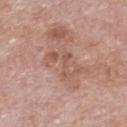Captured during whole-body skin photography for melanoma surveillance; the lesion was not biopsied. A 15 mm close-up tile from a total-body photography series done for melanoma screening. The subject is a male roughly 55 years of age. Located on the chest. Automated image analysis of the tile measured a lesion area of about 6 mm², an eccentricity of roughly 0.8, and a shape-asymmetry score of about 0.75 (0 = symmetric). The software also gave a color-variation rating of about 1/10 and peripheral color asymmetry of about 0. And it measured a classifier nevus-likeness of about 0/100 and a detector confidence of about 100 out of 100 that the crop contains a lesion. The tile uses white-light illumination. About 4 mm across.